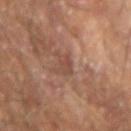biopsy status — total-body-photography surveillance lesion; no biopsy | subject — male, aged approximately 65 | image — total-body-photography crop, ~15 mm field of view | anatomic site — the arm | image-analysis metrics — a footprint of about 3.5 mm², a shape eccentricity near 0.8, and a symmetry-axis asymmetry near 0.25; a lesion color around L≈45 a*≈21 b*≈26 in CIELAB, a lesion–skin lightness drop of about 6, and a normalized lesion–skin contrast near 5; a border-irregularity rating of about 2.5/10 and a color-variation rating of about 2/10; a nevus-likeness score of about 0/100 and a detector confidence of about 85 out of 100 that the crop contains a lesion | lighting — cross-polarized | size — ≈2.5 mm.